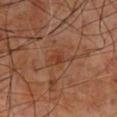Imaged during a routine full-body skin examination; the lesion was not biopsied and no histopathology is available. The tile uses cross-polarized illumination. A close-up tile cropped from a whole-body skin photograph, about 15 mm across. The lesion is on the mid back. The patient is a male roughly 60 years of age. Approximately 3.5 mm at its widest.